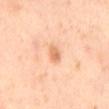Findings:
- follow-up: total-body-photography surveillance lesion; no biopsy
- site: the back
- lesion size: about 2.5 mm
- illumination: cross-polarized illumination
- image: ~15 mm crop, total-body skin-cancer survey
- patient: male, aged approximately 65